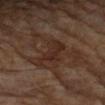From the left forearm. Imaged with cross-polarized lighting. About 4.5 mm across. This image is a 15 mm lesion crop taken from a total-body photograph. The subject is a male aged 83 or older.